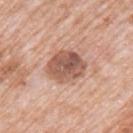Acquisition and patient details:
The recorded lesion diameter is about 4.5 mm. A 15 mm crop from a total-body photograph taken for skin-cancer surveillance. Located on the left upper arm. The patient is a male aged 78–82. Imaged with white-light lighting.